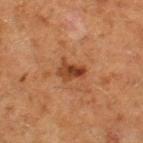Notes:
- notes · no biopsy performed (imaged during a skin exam)
- body site · the left thigh
- illumination · cross-polarized illumination
- acquisition · total-body-photography crop, ~15 mm field of view
- subject · female, aged 48 to 52
- lesion size · about 3 mm